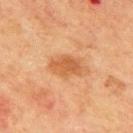Impression: Recorded during total-body skin imaging; not selected for excision or biopsy.